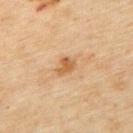patient: male, aged 43–47
automated metrics: an area of roughly 4 mm², an outline eccentricity of about 0.7 (0 = round, 1 = elongated), and a symmetry-axis asymmetry near 0.25; border irregularity of about 2.5 on a 0–10 scale; a lesion-detection confidence of about 100/100
lighting: cross-polarized
image: ~15 mm crop, total-body skin-cancer survey
lesion diameter: about 2.5 mm
location: the upper back